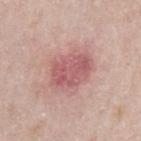follow-up = catalogued during a skin exam; not biopsied.